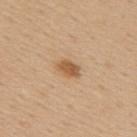notes=imaged on a skin check; not biopsied
tile lighting=white-light illumination
image=~15 mm tile from a whole-body skin photo
body site=the upper back
patient=female, aged 43 to 47